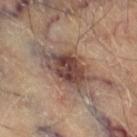Recorded during total-body skin imaging; not selected for excision or biopsy.
Captured under cross-polarized illumination.
The lesion is on the right thigh.
Automated image analysis of the tile measured about 13 CIELAB-L* units darker than the surrounding skin and a lesion-to-skin contrast of about 10.5 (normalized; higher = more distinct). The analysis additionally found a border-irregularity index near 3.5/10, internal color variation of about 6.5 on a 0–10 scale, and radial color variation of about 2. The analysis additionally found an automated nevus-likeness rating near 80 out of 100 and lesion-presence confidence of about 100/100.
Cropped from a total-body skin-imaging series; the visible field is about 15 mm.
The subject is a male roughly 70 years of age.
Measured at roughly 6 mm in maximum diameter.